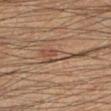<lesion>
  <biopsy_status>not biopsied; imaged during a skin examination</biopsy_status>
  <lesion_size>
    <long_diameter_mm_approx>5.0</long_diameter_mm_approx>
  </lesion_size>
  <image>
    <source>total-body photography crop</source>
    <field_of_view_mm>15</field_of_view_mm>
  </image>
  <lighting>cross-polarized</lighting>
  <patient>
    <sex>male</sex>
    <age_approx>40</age_approx>
  </patient>
  <site>leg</site>
  <automated_metrics>
    <area_mm2_approx>6.0</area_mm2_approx>
    <eccentricity>0.95</eccentricity>
    <shape_asymmetry>0.4</shape_asymmetry>
    <cielab_L>43</cielab_L>
    <cielab_a>16</cielab_a>
    <cielab_b>26</cielab_b>
    <vs_skin_darker_L>7.0</vs_skin_darker_L>
  </automated_metrics>
</lesion>